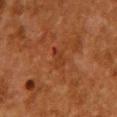Imaged during a routine full-body skin examination; the lesion was not biopsied and no histopathology is available.
A female subject, aged 48–52.
A lesion tile, about 15 mm wide, cut from a 3D total-body photograph.
The lesion is on the chest.